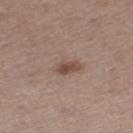Captured during whole-body skin photography for melanoma surveillance; the lesion was not biopsied. A male patient aged 53 to 57. Captured under white-light illumination. Approximately 3.5 mm at its widest. A region of skin cropped from a whole-body photographic capture, roughly 15 mm wide. The lesion-visualizer software estimated a shape-asymmetry score of about 0.3 (0 = symmetric). The software also gave a classifier nevus-likeness of about 85/100 and lesion-presence confidence of about 100/100. The lesion is located on the leg.